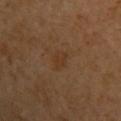notes — catalogued during a skin exam; not biopsied | image-analysis metrics — roughly 5 lightness units darker than nearby skin and a normalized border contrast of about 5.5; a classifier nevus-likeness of about 10/100 and lesion-presence confidence of about 100/100 | image source — ~15 mm crop, total-body skin-cancer survey | lesion size — about 2.5 mm | patient — female, aged approximately 50 | site — the left upper arm.